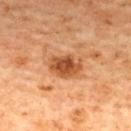Assessment:
Part of a total-body skin-imaging series; this lesion was reviewed on a skin check and was not flagged for biopsy.
Acquisition and patient details:
Approximately 4 mm at its widest. The total-body-photography lesion software estimated a lesion area of about 9.5 mm². The software also gave a classifier nevus-likeness of about 90/100. Located on the upper back. A roughly 15 mm field-of-view crop from a total-body skin photograph. Captured under cross-polarized illumination. The patient is a female aged 58 to 62.